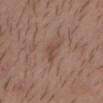biopsy status = catalogued during a skin exam; not biopsied
TBP lesion metrics = a lesion–skin lightness drop of about 7; a classifier nevus-likeness of about 0/100 and a detector confidence of about 100 out of 100 that the crop contains a lesion
size = ≈3 mm
tile lighting = white-light
image = total-body-photography crop, ~15 mm field of view
site = the head or neck
subject = male, in their 30s